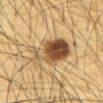No biopsy was performed on this lesion — it was imaged during a full skin examination and was not determined to be concerning.
Measured at roughly 6.5 mm in maximum diameter.
Cropped from a total-body skin-imaging series; the visible field is about 15 mm.
The lesion is on the abdomen.
This is a cross-polarized tile.
Automated tile analysis of the lesion measured a footprint of about 17 mm² and two-axis asymmetry of about 0.25. It also reported a nevus-likeness score of about 95/100.
A male subject, approximately 65 years of age.